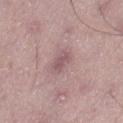The lesion was tiled from a total-body skin photograph and was not biopsied.
On the left thigh.
A lesion tile, about 15 mm wide, cut from a 3D total-body photograph.
A male subject aged 53–57.
The tile uses white-light illumination.